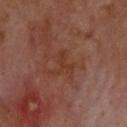Case summary:
• biopsy status: imaged on a skin check; not biopsied
• anatomic site: the upper back
• lighting: cross-polarized
• lesion size: ≈3 mm
• patient: male, aged 68 to 72
• image: 15 mm crop, total-body photography
• TBP lesion metrics: a footprint of about 3.5 mm² and a shape-asymmetry score of about 0.65 (0 = symmetric); an average lesion color of about L≈35 a*≈22 b*≈28 (CIELAB) and a lesion-to-skin contrast of about 5 (normalized; higher = more distinct)